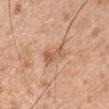Imaged during a routine full-body skin examination; the lesion was not biopsied and no histopathology is available.
The total-body-photography lesion software estimated an area of roughly 4.5 mm², an outline eccentricity of about 0.9 (0 = round, 1 = elongated), and a symmetry-axis asymmetry near 0.3. It also reported a mean CIELAB color near L≈59 a*≈21 b*≈34, a lesion–skin lightness drop of about 10, and a normalized lesion–skin contrast near 6.5. And it measured a within-lesion color-variation index near 4/10 and peripheral color asymmetry of about 1.5.
The patient is a male roughly 45 years of age.
On the chest.
Imaged with white-light lighting.
The lesion's longest dimension is about 3.5 mm.
Cropped from a total-body skin-imaging series; the visible field is about 15 mm.